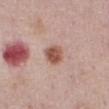The lesion was photographed on a routine skin check and not biopsied; there is no pathology result. The lesion is located on the abdomen. The total-body-photography lesion software estimated a lesion area of about 5.5 mm² and a shape eccentricity near 0.2. The tile uses white-light illumination. The lesion's longest dimension is about 2.5 mm. The subject is a male aged around 75. A 15 mm crop from a total-body photograph taken for skin-cancer surveillance.